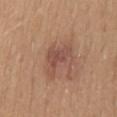Imaged during a routine full-body skin examination; the lesion was not biopsied and no histopathology is available. Cropped from a whole-body photographic skin survey; the tile spans about 15 mm. Located on the back. The patient is a female aged approximately 55. About 5 mm across. The tile uses white-light illumination. The total-body-photography lesion software estimated a footprint of about 10 mm², a shape eccentricity near 0.75, and a symmetry-axis asymmetry near 0.45. The analysis additionally found a lesion color around L≈50 a*≈21 b*≈27 in CIELAB, about 8 CIELAB-L* units darker than the surrounding skin, and a normalized lesion–skin contrast near 6.5. And it measured a border-irregularity rating of about 5.5/10, internal color variation of about 3.5 on a 0–10 scale, and a peripheral color-asymmetry measure near 1. The analysis additionally found an automated nevus-likeness rating near 10 out of 100.